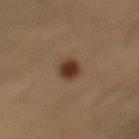Located on the mid back. A male subject, roughly 55 years of age. This is a cross-polarized tile. A close-up tile cropped from a whole-body skin photograph, about 15 mm across.